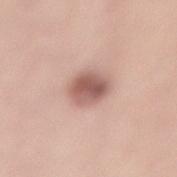The lesion was photographed on a routine skin check and not biopsied; there is no pathology result.
The tile uses white-light illumination.
The recorded lesion diameter is about 3.5 mm.
A lesion tile, about 15 mm wide, cut from a 3D total-body photograph.
A male subject about 35 years old.
On the lower back.
An algorithmic analysis of the crop reported a lesion area of about 9 mm², an outline eccentricity of about 0.3 (0 = round, 1 = elongated), and two-axis asymmetry of about 0.1. The analysis additionally found a lesion color around L≈58 a*≈21 b*≈24 in CIELAB, about 14 CIELAB-L* units darker than the surrounding skin, and a normalized border contrast of about 9. And it measured a color-variation rating of about 5/10.